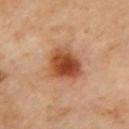{"biopsy_status": "not biopsied; imaged during a skin examination", "site": "mid back", "lesion_size": {"long_diameter_mm_approx": 4.0}, "automated_metrics": {"cielab_L": 50, "cielab_a": 27, "cielab_b": 37, "vs_skin_darker_L": 15.0, "vs_skin_contrast_norm": 10.5, "border_irregularity_0_10": 2.5}, "image": {"source": "total-body photography crop", "field_of_view_mm": 15}, "lighting": "cross-polarized"}